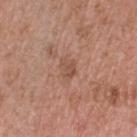Q: Is there a histopathology result?
A: imaged on a skin check; not biopsied
Q: Lesion location?
A: the head or neck
Q: What is the lesion's diameter?
A: about 2.5 mm
Q: What are the patient's age and sex?
A: male, aged around 50
Q: Illumination type?
A: white-light illumination
Q: Automated lesion metrics?
A: a mean CIELAB color near L≈51 a*≈21 b*≈29, a lesion–skin lightness drop of about 7, and a normalized border contrast of about 5.5; a border-irregularity rating of about 3/10 and a peripheral color-asymmetry measure near 0.5
Q: What is the imaging modality?
A: total-body-photography crop, ~15 mm field of view